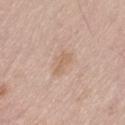No biopsy was performed on this lesion — it was imaged during a full skin examination and was not determined to be concerning. A roughly 15 mm field-of-view crop from a total-body skin photograph. A male patient in their mid- to late 70s. The lesion-visualizer software estimated roughly 7 lightness units darker than nearby skin and a normalized border contrast of about 5.5. It also reported a border-irregularity index near 3.5/10, internal color variation of about 1 on a 0–10 scale, and peripheral color asymmetry of about 0.5. And it measured a classifier nevus-likeness of about 0/100 and a detector confidence of about 100 out of 100 that the crop contains a lesion. This is a white-light tile. The lesion's longest dimension is about 3 mm. Located on the leg.